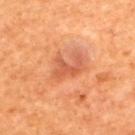The lesion was tiled from a total-body skin photograph and was not biopsied.
Automated image analysis of the tile measured a border-irregularity rating of about 7.5/10 and a peripheral color-asymmetry measure near 0. And it measured a nevus-likeness score of about 5/100.
Measured at roughly 3 mm in maximum diameter.
The tile uses cross-polarized illumination.
The lesion is on the upper back.
Cropped from a total-body skin-imaging series; the visible field is about 15 mm.
The subject is a female in their mid-50s.